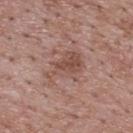Imaged during a routine full-body skin examination; the lesion was not biopsied and no histopathology is available.
Imaged with white-light lighting.
A male patient aged approximately 70.
Automated tile analysis of the lesion measured a lesion area of about 11 mm², an eccentricity of roughly 0.75, and a shape-asymmetry score of about 0.5 (0 = symmetric). The software also gave an average lesion color of about L≈50 a*≈20 b*≈25 (CIELAB) and a normalized lesion–skin contrast near 6. The software also gave a nevus-likeness score of about 0/100 and a lesion-detection confidence of about 100/100.
A region of skin cropped from a whole-body photographic capture, roughly 15 mm wide.
The lesion's longest dimension is about 4.5 mm.
From the upper back.